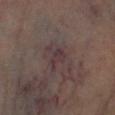{"biopsy_status": "not biopsied; imaged during a skin examination", "image": {"source": "total-body photography crop", "field_of_view_mm": 15}, "lighting": "cross-polarized", "patient": {"sex": "female", "age_approx": 50}, "lesion_size": {"long_diameter_mm_approx": 3.0}, "site": "right lower leg"}